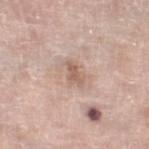Notes:
* biopsy status — no biopsy performed (imaged during a skin exam)
* tile lighting — white-light
* location — the right lower leg
* acquisition — ~15 mm crop, total-body skin-cancer survey
* patient — female, aged 58 to 62